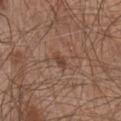No biopsy was performed on this lesion — it was imaged during a full skin examination and was not determined to be concerning.
The subject is a male aged approximately 65.
Imaged with white-light lighting.
This image is a 15 mm lesion crop taken from a total-body photograph.
An algorithmic analysis of the crop reported a mean CIELAB color near L≈44 a*≈19 b*≈27, roughly 7 lightness units darker than nearby skin, and a normalized lesion–skin contrast near 6. The analysis additionally found a border-irregularity rating of about 3/10, internal color variation of about 1.5 on a 0–10 scale, and peripheral color asymmetry of about 0.5.
Approximately 2.5 mm at its widest.
From the chest.